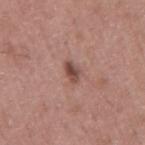Captured under white-light illumination. An algorithmic analysis of the crop reported a lesion area of about 3.5 mm², an outline eccentricity of about 0.8 (0 = round, 1 = elongated), and a shape-asymmetry score of about 0.25 (0 = symmetric). Cropped from a whole-body photographic skin survey; the tile spans about 15 mm. Located on the mid back. A male patient, aged approximately 60. The recorded lesion diameter is about 2.5 mm.